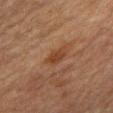Imaged during a routine full-body skin examination; the lesion was not biopsied and no histopathology is available. On the abdomen. A 15 mm close-up extracted from a 3D total-body photography capture. The patient is a male about 80 years old.